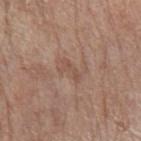Q: Was this lesion biopsied?
A: total-body-photography surveillance lesion; no biopsy
Q: Illumination type?
A: white-light illumination
Q: Patient demographics?
A: female, about 75 years old
Q: How large is the lesion?
A: about 2.5 mm
Q: Where on the body is the lesion?
A: the left forearm
Q: Automated lesion metrics?
A: an average lesion color of about L≈51 a*≈18 b*≈26 (CIELAB) and roughly 6 lightness units darker than nearby skin; lesion-presence confidence of about 100/100
Q: What is the imaging modality?
A: ~15 mm crop, total-body skin-cancer survey